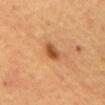notes: catalogued during a skin exam; not biopsied
lesion diameter: about 3 mm
body site: the abdomen
imaging modality: ~15 mm crop, total-body skin-cancer survey
lighting: cross-polarized illumination
subject: female
TBP lesion metrics: a border-irregularity index near 2/10, a within-lesion color-variation index near 4/10, and a peripheral color-asymmetry measure near 1.5; a classifier nevus-likeness of about 95/100